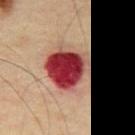Clinical summary:
Captured under cross-polarized illumination. Longest diameter approximately 6 mm. The lesion is located on the front of the torso. A male subject aged 43 to 47. A roughly 15 mm field-of-view crop from a total-body skin photograph.